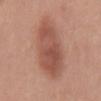Assessment:
Part of a total-body skin-imaging series; this lesion was reviewed on a skin check and was not flagged for biopsy.
Background:
Longest diameter approximately 9 mm. A female patient, in their 40s. Automated image analysis of the tile measured an area of roughly 25 mm² and two-axis asymmetry of about 0.15. And it measured an average lesion color of about L≈52 a*≈24 b*≈28 (CIELAB) and about 11 CIELAB-L* units darker than the surrounding skin. The analysis additionally found a border-irregularity rating of about 2.5/10 and internal color variation of about 4 on a 0–10 scale. And it measured an automated nevus-likeness rating near 95 out of 100 and lesion-presence confidence of about 100/100. A close-up tile cropped from a whole-body skin photograph, about 15 mm across. The lesion is on the mid back. Captured under white-light illumination.